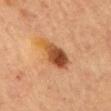Clinical impression:
No biopsy was performed on this lesion — it was imaged during a full skin examination and was not determined to be concerning.
Background:
The lesion's longest dimension is about 4.5 mm. The patient is a female about 60 years old. The lesion is on the right upper arm. A roughly 15 mm field-of-view crop from a total-body skin photograph. Automated image analysis of the tile measured a lesion area of about 12 mm², an outline eccentricity of about 0.7 (0 = round, 1 = elongated), and a symmetry-axis asymmetry near 0.25. The analysis additionally found a nevus-likeness score of about 95/100 and lesion-presence confidence of about 100/100.